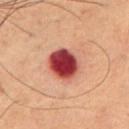Captured during whole-body skin photography for melanoma surveillance; the lesion was not biopsied. A male patient, aged 68–72. The lesion is on the chest. Cropped from a whole-body photographic skin survey; the tile spans about 15 mm. Measured at roughly 4 mm in maximum diameter. The tile uses cross-polarized illumination.